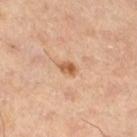Clinical summary: The subject is a male roughly 55 years of age. This is a cross-polarized tile. A close-up tile cropped from a whole-body skin photograph, about 15 mm across. Longest diameter approximately 2.5 mm. Located on the right thigh. An algorithmic analysis of the crop reported an area of roughly 3.5 mm² and a symmetry-axis asymmetry near 0.3.